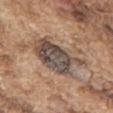Clinical impression: Part of a total-body skin-imaging series; this lesion was reviewed on a skin check and was not flagged for biopsy. Image and clinical context: A male subject, aged 73 to 77. Automated image analysis of the tile measured a mean CIELAB color near L≈48 a*≈13 b*≈23 and about 13 CIELAB-L* units darker than the surrounding skin. The analysis additionally found a border-irregularity rating of about 3.5/10 and a color-variation rating of about 7/10. A close-up tile cropped from a whole-body skin photograph, about 15 mm across. On the front of the torso. Imaged with white-light lighting. Longest diameter approximately 6.5 mm.